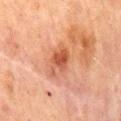notes: no biopsy performed (imaged during a skin exam)
location: the abdomen
automated metrics: a mean CIELAB color near L≈44 a*≈25 b*≈30 and a normalized border contrast of about 8; a peripheral color-asymmetry measure near 1; a nevus-likeness score of about 40/100 and a detector confidence of about 100 out of 100 that the crop contains a lesion
tile lighting: cross-polarized illumination
imaging modality: ~15 mm crop, total-body skin-cancer survey
patient: male, aged 63–67
lesion size: ~4 mm (longest diameter)